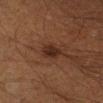* workup — total-body-photography surveillance lesion; no biopsy
* patient — male, approximately 60 years of age
* image — 15 mm crop, total-body photography
* body site — the right lower leg